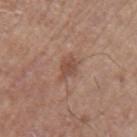Part of a total-body skin-imaging series; this lesion was reviewed on a skin check and was not flagged for biopsy. A male subject, aged around 65. A close-up tile cropped from a whole-body skin photograph, about 15 mm across. On the arm.